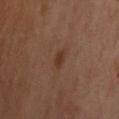Recorded during total-body skin imaging; not selected for excision or biopsy. A male subject aged approximately 45. Cropped from a whole-body photographic skin survey; the tile spans about 15 mm. Imaged with cross-polarized lighting. Automated image analysis of the tile measured roughly 7 lightness units darker than nearby skin and a lesion-to-skin contrast of about 7.5 (normalized; higher = more distinct). And it measured a classifier nevus-likeness of about 90/100 and a detector confidence of about 100 out of 100 that the crop contains a lesion. Measured at roughly 2 mm in maximum diameter. On the upper back.